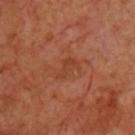Part of a total-body skin-imaging series; this lesion was reviewed on a skin check and was not flagged for biopsy.
About 3 mm across.
Located on the chest.
A male patient aged 68 to 72.
A 15 mm close-up extracted from a 3D total-body photography capture.
The lesion-visualizer software estimated a lesion color around L≈40 a*≈26 b*≈33 in CIELAB, about 5 CIELAB-L* units darker than the surrounding skin, and a lesion-to-skin contrast of about 5 (normalized; higher = more distinct). The analysis additionally found an automated nevus-likeness rating near 0 out of 100.